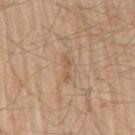Clinical impression:
The lesion was tiled from a total-body skin photograph and was not biopsied.
Context:
From the right upper arm. The patient is a male roughly 80 years of age. Automated image analysis of the tile measured a border-irregularity index near 4.5/10, a within-lesion color-variation index near 0/10, and a peripheral color-asymmetry measure near 0. The analysis additionally found a classifier nevus-likeness of about 0/100. This image is a 15 mm lesion crop taken from a total-body photograph. The lesion's longest dimension is about 3 mm. Captured under white-light illumination.